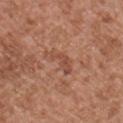biopsy status = imaged on a skin check; not biopsied.